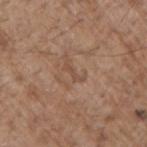Measured at roughly 2.5 mm in maximum diameter. A close-up tile cropped from a whole-body skin photograph, about 15 mm across. From the right upper arm. The subject is a male about 70 years old. Captured under white-light illumination.